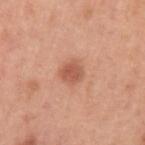The lesion was photographed on a routine skin check and not biopsied; there is no pathology result. Captured under white-light illumination. About 3 mm across. From the left upper arm. A male patient aged 48–52. A close-up tile cropped from a whole-body skin photograph, about 15 mm across.